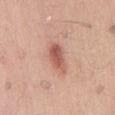Impression: The lesion was tiled from a total-body skin photograph and was not biopsied. Clinical summary: About 3.5 mm across. The lesion is located on the back. A 15 mm close-up extracted from a 3D total-body photography capture. A male subject, aged approximately 55. Automated tile analysis of the lesion measured an eccentricity of roughly 0.8 and a symmetry-axis asymmetry near 0.2. The software also gave a lesion color around L≈57 a*≈25 b*≈28 in CIELAB and a normalized lesion–skin contrast near 8. The analysis additionally found a color-variation rating of about 4.5/10 and a peripheral color-asymmetry measure near 1.5. The analysis additionally found a lesion-detection confidence of about 100/100.